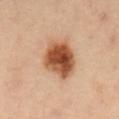Assessment:
The lesion was tiled from a total-body skin photograph and was not biopsied.
Acquisition and patient details:
This image is a 15 mm lesion crop taken from a total-body photograph. The patient is a male aged around 40. From the mid back.